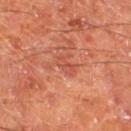Imaged during a routine full-body skin examination; the lesion was not biopsied and no histopathology is available. The lesion's longest dimension is about 2.5 mm. Cropped from a whole-body photographic skin survey; the tile spans about 15 mm. The lesion is located on the left thigh. The subject is a male aged 63–67.